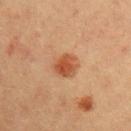Case summary:
- notes · catalogued during a skin exam; not biopsied
- patient · male, aged approximately 40
- imaging modality · total-body-photography crop, ~15 mm field of view
- lesion size · ≈3.5 mm
- location · the right upper arm